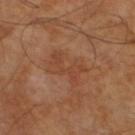biopsy_status: not biopsied; imaged during a skin examination
image:
  source: total-body photography crop
  field_of_view_mm: 15
lesion_size:
  long_diameter_mm_approx: 4.5
site: leg
patient:
  sex: male
  age_approx: 65
automated_metrics:
  area_mm2_approx: 6.5
  eccentricity: 0.9
  shape_asymmetry: 0.55
  lesion_detection_confidence_0_100: 100
lighting: cross-polarized